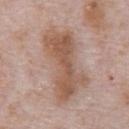Captured during whole-body skin photography for melanoma surveillance; the lesion was not biopsied. Located on the chest. A 15 mm crop from a total-body photograph taken for skin-cancer surveillance. A male subject roughly 70 years of age. The tile uses white-light illumination. Approximately 9.5 mm at its widest.